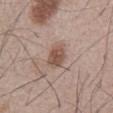Case summary:
- follow-up · imaged on a skin check; not biopsied
- acquisition · 15 mm crop, total-body photography
- diameter · ~3.5 mm (longest diameter)
- subject · male, approximately 55 years of age
- illumination · white-light illumination